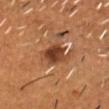Findings:
- follow-up: catalogued during a skin exam; not biopsied
- subject: male, approximately 55 years of age
- diameter: about 3.5 mm
- illumination: cross-polarized illumination
- image: 15 mm crop, total-body photography
- automated metrics: about 12 CIELAB-L* units darker than the surrounding skin; a lesion-detection confidence of about 100/100
- anatomic site: the head or neck